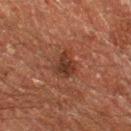Impression:
This lesion was catalogued during total-body skin photography and was not selected for biopsy.
Acquisition and patient details:
The lesion is on the left thigh. A 15 mm close-up tile from a total-body photography series done for melanoma screening. A male patient, aged approximately 60.